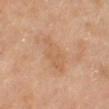location — the right lower leg | subject — female, aged around 70 | TBP lesion metrics — an area of roughly 9.5 mm², an outline eccentricity of about 0.95 (0 = round, 1 = elongated), and a symmetry-axis asymmetry near 0.3; a detector confidence of about 100 out of 100 that the crop contains a lesion | acquisition — ~15 mm tile from a whole-body skin photo | lesion diameter — ≈6 mm | tile lighting — cross-polarized illumination.